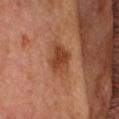follow-up: no biopsy performed (imaged during a skin exam); subject: male, aged 53–57; image source: total-body-photography crop, ~15 mm field of view; body site: the head or neck; lesion diameter: ≈4 mm; illumination: cross-polarized.